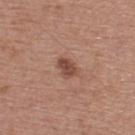| field | value |
|---|---|
| biopsy status | total-body-photography surveillance lesion; no biopsy |
| location | the upper back |
| automated metrics | an average lesion color of about L≈47 a*≈22 b*≈27 (CIELAB) and a lesion-to-skin contrast of about 8.5 (normalized; higher = more distinct); a lesion-detection confidence of about 100/100 |
| diameter | about 2.5 mm |
| acquisition | total-body-photography crop, ~15 mm field of view |
| illumination | white-light |
| subject | male, about 55 years old |